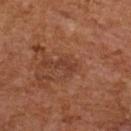Captured during whole-body skin photography for melanoma surveillance; the lesion was not biopsied.
Imaged with cross-polarized lighting.
A female patient, approximately 60 years of age.
Longest diameter approximately 2.5 mm.
This image is a 15 mm lesion crop taken from a total-body photograph.
The lesion is on the back.